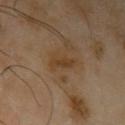| field | value |
|---|---|
| follow-up | no biopsy performed (imaged during a skin exam) |
| image source | ~15 mm tile from a whole-body skin photo |
| size | ~3.5 mm (longest diameter) |
| subject | male, in their mid-60s |
| site | the left upper arm |
| illumination | cross-polarized |